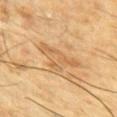Captured during whole-body skin photography for melanoma surveillance; the lesion was not biopsied.
From the chest.
A male subject, in their mid- to late 80s or older.
Automated tile analysis of the lesion measured an area of roughly 10 mm², a shape eccentricity near 0.85, and a symmetry-axis asymmetry near 0.55.
Approximately 5.5 mm at its widest.
The tile uses cross-polarized illumination.
A lesion tile, about 15 mm wide, cut from a 3D total-body photograph.